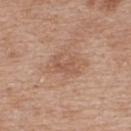Assessment:
Captured during whole-body skin photography for melanoma surveillance; the lesion was not biopsied.
Image and clinical context:
The lesion-visualizer software estimated an area of roughly 4 mm², an outline eccentricity of about 0.75 (0 = round, 1 = elongated), and a shape-asymmetry score of about 0.5 (0 = symmetric). The software also gave a lesion–skin lightness drop of about 7 and a normalized border contrast of about 5. The analysis additionally found a nevus-likeness score of about 0/100 and lesion-presence confidence of about 100/100. A region of skin cropped from a whole-body photographic capture, roughly 15 mm wide. This is a white-light tile. The lesion is on the upper back. The subject is a female aged 38 to 42. The recorded lesion diameter is about 3 mm.